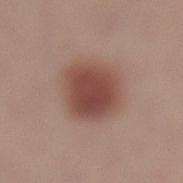This lesion was catalogued during total-body skin photography and was not selected for biopsy. A female subject aged approximately 25. From the left lower leg. This image is a 15 mm lesion crop taken from a total-body photograph.